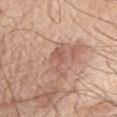Captured during whole-body skin photography for melanoma surveillance; the lesion was not biopsied. A close-up tile cropped from a whole-body skin photograph, about 15 mm across. On the chest. A male subject in their mid-60s. This is a white-light tile.